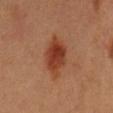Context:
Measured at roughly 6 mm in maximum diameter. The subject is a male in their 60s. Located on the abdomen. The tile uses cross-polarized illumination. This image is a 15 mm lesion crop taken from a total-body photograph.